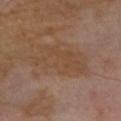{"biopsy_status": "not biopsied; imaged during a skin examination", "lighting": "cross-polarized", "site": "right forearm", "image": {"source": "total-body photography crop", "field_of_view_mm": 15}, "patient": {"sex": "male", "age_approx": 55}, "lesion_size": {"long_diameter_mm_approx": 7.0}, "automated_metrics": {"nevus_likeness_0_100": 0, "lesion_detection_confidence_0_100": 100}}